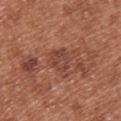{
  "biopsy_status": "not biopsied; imaged during a skin examination",
  "image": {
    "source": "total-body photography crop",
    "field_of_view_mm": 15
  },
  "patient": {
    "sex": "female",
    "age_approx": 40
  },
  "site": "back",
  "automated_metrics": {
    "area_mm2_approx": 6.0,
    "eccentricity": 0.45,
    "shape_asymmetry": 0.25
  },
  "lighting": "white-light",
  "lesion_size": {
    "long_diameter_mm_approx": 3.0
  }
}